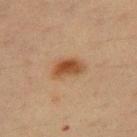<lesion>
  <biopsy_status>not biopsied; imaged during a skin examination</biopsy_status>
  <image>
    <source>total-body photography crop</source>
    <field_of_view_mm>15</field_of_view_mm>
  </image>
  <automated_metrics>
    <cielab_L>39</cielab_L>
    <cielab_a>18</cielab_a>
    <cielab_b>31</cielab_b>
    <vs_skin_darker_L>10.0</vs_skin_darker_L>
    <vs_skin_contrast_norm>9.5</vs_skin_contrast_norm>
    <border_irregularity_0_10>3.0</border_irregularity_0_10>
    <color_variation_0_10>4.0</color_variation_0_10>
    <peripheral_color_asymmetry>1.0</peripheral_color_asymmetry>
  </automated_metrics>
  <site>right thigh</site>
  <patient>
    <sex>female</sex>
    <age_approx>55</age_approx>
  </patient>
  <lesion_size>
    <long_diameter_mm_approx>3.5</long_diameter_mm_approx>
  </lesion_size>
</lesion>